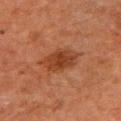patient:
  sex: male
  age_approx: 60
image:
  source: total-body photography crop
  field_of_view_mm: 15
site: left upper arm